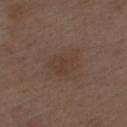This lesion was catalogued during total-body skin photography and was not selected for biopsy.
The recorded lesion diameter is about 4 mm.
Located on the upper back.
Captured under white-light illumination.
A 15 mm crop from a total-body photograph taken for skin-cancer surveillance.
A male subject aged approximately 70.
The lesion-visualizer software estimated a lesion area of about 9 mm² and an outline eccentricity of about 0.7 (0 = round, 1 = elongated). The software also gave border irregularity of about 4 on a 0–10 scale, a within-lesion color-variation index near 2/10, and radial color variation of about 0.5.